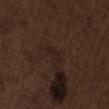workup = imaged on a skin check; not biopsied
patient = male, approximately 70 years of age
imaging modality = total-body-photography crop, ~15 mm field of view
illumination = white-light illumination
TBP lesion metrics = an area of roughly 2 mm² and two-axis asymmetry of about 0.4; a nevus-likeness score of about 0/100 and a detector confidence of about 100 out of 100 that the crop contains a lesion
site = the lower back